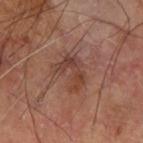Context: The tile uses cross-polarized illumination. An algorithmic analysis of the crop reported a lesion area of about 8.5 mm², an outline eccentricity of about 0.7 (0 = round, 1 = elongated), and a symmetry-axis asymmetry near 0.5. The software also gave an average lesion color of about L≈41 a*≈22 b*≈26 (CIELAB), a lesion–skin lightness drop of about 8, and a normalized lesion–skin contrast near 6.5. It also reported a border-irregularity index near 6.5/10 and internal color variation of about 7 on a 0–10 scale. It also reported an automated nevus-likeness rating near 0 out of 100 and a lesion-detection confidence of about 100/100. A male subject in their mid- to late 60s. Located on the left forearm. A close-up tile cropped from a whole-body skin photograph, about 15 mm across. The recorded lesion diameter is about 4 mm.